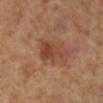| feature | finding |
|---|---|
| biopsy status | no biopsy performed (imaged during a skin exam) |
| image source | ~15 mm crop, total-body skin-cancer survey |
| anatomic site | the right lower leg |
| subject | female, aged 68 to 72 |
| diameter | about 4.5 mm |
| lighting | cross-polarized |